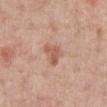| key | value |
|---|---|
| biopsy status | imaged on a skin check; not biopsied |
| patient | male, approximately 60 years of age |
| site | the abdomen |
| TBP lesion metrics | two-axis asymmetry of about 0.45 |
| tile lighting | white-light illumination |
| image source | total-body-photography crop, ~15 mm field of view |